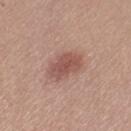This lesion was catalogued during total-body skin photography and was not selected for biopsy. The lesion's longest dimension is about 4 mm. The lesion is located on the left thigh. Automated tile analysis of the lesion measured an area of roughly 8.5 mm², a shape eccentricity near 0.7, and two-axis asymmetry of about 0.2. It also reported a mean CIELAB color near L≈52 a*≈22 b*≈25 and a normalized lesion–skin contrast near 7. And it measured a border-irregularity rating of about 2.5/10, a color-variation rating of about 4/10, and radial color variation of about 1.5. A 15 mm close-up tile from a total-body photography series done for melanoma screening. Captured under white-light illumination. A female subject aged around 30.